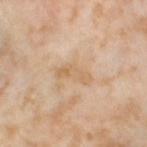Imaged during a routine full-body skin examination; the lesion was not biopsied and no histopathology is available.